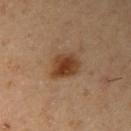{
  "biopsy_status": "not biopsied; imaged during a skin examination",
  "image": {
    "source": "total-body photography crop",
    "field_of_view_mm": 15
  },
  "lesion_size": {
    "long_diameter_mm_approx": 4.0
  },
  "site": "right upper arm",
  "patient": {
    "sex": "male",
    "age_approx": 55
  },
  "lighting": "cross-polarized"
}